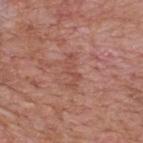| key | value |
|---|---|
| follow-up | imaged on a skin check; not biopsied |
| patient | male, approximately 65 years of age |
| site | the mid back |
| size | ≈3.5 mm |
| image source | total-body-photography crop, ~15 mm field of view |